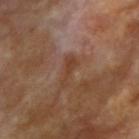Captured during whole-body skin photography for melanoma surveillance; the lesion was not biopsied.
Measured at roughly 3.5 mm in maximum diameter.
The lesion is located on the left upper arm.
Captured under cross-polarized illumination.
A female patient aged 73–77.
Cropped from a total-body skin-imaging series; the visible field is about 15 mm.
Automated tile analysis of the lesion measured an area of roughly 3 mm², an eccentricity of roughly 0.95, and a shape-asymmetry score of about 0.6 (0 = symmetric). The software also gave border irregularity of about 6.5 on a 0–10 scale, a within-lesion color-variation index near 1/10, and a peripheral color-asymmetry measure near 0.